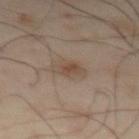Part of a total-body skin-imaging series; this lesion was reviewed on a skin check and was not flagged for biopsy.
Automated tile analysis of the lesion measured a footprint of about 5 mm², an eccentricity of roughly 0.75, and a shape-asymmetry score of about 0.3 (0 = symmetric). It also reported radial color variation of about 0.5. The analysis additionally found a nevus-likeness score of about 50/100 and a lesion-detection confidence of about 100/100.
Captured under cross-polarized illumination.
The recorded lesion diameter is about 3.5 mm.
The lesion is located on the left thigh.
A male patient, in their mid- to late 50s.
A roughly 15 mm field-of-view crop from a total-body skin photograph.